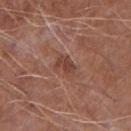The lesion was photographed on a routine skin check and not biopsied; there is no pathology result. A male subject, aged 63 to 67. The tile uses white-light illumination. A 15 mm close-up tile from a total-body photography series done for melanoma screening. The lesion is located on the arm.